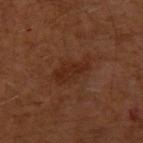Imaged during a routine full-body skin examination; the lesion was not biopsied and no histopathology is available. Located on the left upper arm. An algorithmic analysis of the crop reported a nevus-likeness score of about 5/100 and a lesion-detection confidence of about 100/100. A male patient approximately 60 years of age. Imaged with cross-polarized lighting. Measured at roughly 4.5 mm in maximum diameter. A 15 mm close-up tile from a total-body photography series done for melanoma screening.